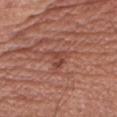The lesion was photographed on a routine skin check and not biopsied; there is no pathology result.
The subject is a male aged 68 to 72.
The lesion is on the right upper arm.
The lesion-visualizer software estimated border irregularity of about 5 on a 0–10 scale, a color-variation rating of about 1.5/10, and a peripheral color-asymmetry measure near 0.5.
This image is a 15 mm lesion crop taken from a total-body photograph.
Approximately 2.5 mm at its widest.
Captured under white-light illumination.